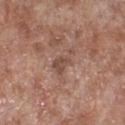Impression:
The lesion was tiled from a total-body skin photograph and was not biopsied.
Acquisition and patient details:
From the leg. The patient is a male in their mid- to late 50s. Cropped from a total-body skin-imaging series; the visible field is about 15 mm.